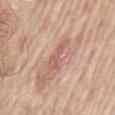Impression:
The lesion was tiled from a total-body skin photograph and was not biopsied.